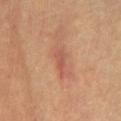{
  "biopsy_status": "not biopsied; imaged during a skin examination",
  "lesion_size": {
    "long_diameter_mm_approx": 4.0
  },
  "automated_metrics": {
    "area_mm2_approx": 4.5,
    "shape_asymmetry": 0.3,
    "border_irregularity_0_10": 4.0,
    "color_variation_0_10": 1.0,
    "peripheral_color_asymmetry": 0.0,
    "nevus_likeness_0_100": 0,
    "lesion_detection_confidence_0_100": 95
  },
  "patient": {
    "sex": "male",
    "age_approx": "85+"
  },
  "lighting": "cross-polarized",
  "site": "front of the torso",
  "image": {
    "source": "total-body photography crop",
    "field_of_view_mm": 15
  }
}